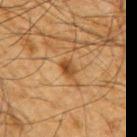Imaged during a routine full-body skin examination; the lesion was not biopsied and no histopathology is available. Automated tile analysis of the lesion measured a footprint of about 3.5 mm², an eccentricity of roughly 0.75, and a shape-asymmetry score of about 0.2 (0 = symmetric). And it measured roughly 9 lightness units darker than nearby skin and a normalized lesion–skin contrast near 8. It also reported an automated nevus-likeness rating near 70 out of 100 and lesion-presence confidence of about 100/100. From the right upper arm. A male patient aged around 65. About 2.5 mm across. A close-up tile cropped from a whole-body skin photograph, about 15 mm across.